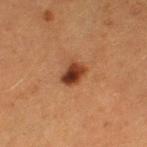| feature | finding |
|---|---|
| notes | imaged on a skin check; not biopsied |
| patient | female, in their 50s |
| anatomic site | the left thigh |
| tile lighting | cross-polarized illumination |
| automated metrics | a footprint of about 5 mm², an eccentricity of roughly 0.75, and two-axis asymmetry of about 0.25; a normalized border contrast of about 12 |
| acquisition | 15 mm crop, total-body photography |